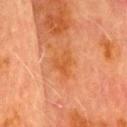  biopsy_status: not biopsied; imaged during a skin examination
  image:
    source: total-body photography crop
    field_of_view_mm: 15
  lighting: cross-polarized
  patient:
    sex: male
    age_approx: 70
  lesion_size:
    long_diameter_mm_approx: 3.0
  automated_metrics:
    area_mm2_approx: 5.0
    eccentricity: 0.65
    shape_asymmetry: 0.5
    color_variation_0_10: 2.5
    peripheral_color_asymmetry: 1.0
    nevus_likeness_0_100: 0
    lesion_detection_confidence_0_100: 100
  site: head or neck